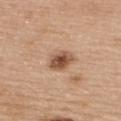<tbp_lesion>
<biopsy_status>not biopsied; imaged during a skin examination</biopsy_status>
<site>upper back</site>
<image>
  <source>total-body photography crop</source>
  <field_of_view_mm>15</field_of_view_mm>
</image>
<automated_metrics>
  <peripheral_color_asymmetry>1.0</peripheral_color_asymmetry>
</automated_metrics>
<lesion_size>
  <long_diameter_mm_approx>3.5</long_diameter_mm_approx>
</lesion_size>
<lighting>white-light</lighting>
<patient>
  <sex>female</sex>
  <age_approx>65</age_approx>
</patient>
</tbp_lesion>